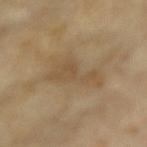workup: imaged on a skin check; not biopsied | location: the left forearm | tile lighting: cross-polarized | subject: female, aged approximately 75 | acquisition: 15 mm crop, total-body photography | image-analysis metrics: an area of roughly 12 mm², a shape eccentricity near 0.9, and a symmetry-axis asymmetry near 0.5; a within-lesion color-variation index near 2/10 and radial color variation of about 0.5; an automated nevus-likeness rating near 0 out of 100 | size: ~6 mm (longest diameter).